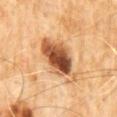Q: Is there a histopathology result?
A: catalogued during a skin exam; not biopsied